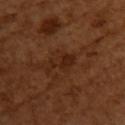Clinical summary: Cropped from a whole-body photographic skin survey; the tile spans about 15 mm. The lesion's longest dimension is about 4 mm. Imaged with cross-polarized lighting. The lesion is on the upper back. The patient is a female in their mid- to late 50s.